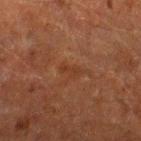This lesion was catalogued during total-body skin photography and was not selected for biopsy.
A 15 mm crop from a total-body photograph taken for skin-cancer surveillance.
The subject is a male aged 58 to 62.
From the right lower leg.
This is a cross-polarized tile.